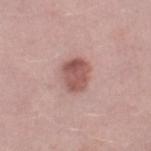- workup: no biopsy performed (imaged during a skin exam)
- diameter: ~3.5 mm (longest diameter)
- tile lighting: white-light
- patient: male, roughly 40 years of age
- anatomic site: the right thigh
- automated lesion analysis: an area of roughly 9 mm² and an outline eccentricity of about 0.55 (0 = round, 1 = elongated); a mean CIELAB color near L≈54 a*≈23 b*≈23, about 13 CIELAB-L* units darker than the surrounding skin, and a normalized lesion–skin contrast near 8.5; a border-irregularity rating of about 1.5/10 and peripheral color asymmetry of about 1.5
- image source: 15 mm crop, total-body photography